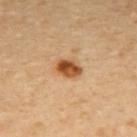The lesion was tiled from a total-body skin photograph and was not biopsied. On the upper back. Measured at roughly 3 mm in maximum diameter. The lesion-visualizer software estimated a mean CIELAB color near L≈53 a*≈24 b*≈41, roughly 16 lightness units darker than nearby skin, and a normalized lesion–skin contrast near 11. It also reported a within-lesion color-variation index near 7/10 and peripheral color asymmetry of about 2.5. The analysis additionally found a classifier nevus-likeness of about 100/100. A female subject, approximately 55 years of age. A 15 mm close-up extracted from a 3D total-body photography capture.